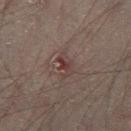Clinical impression: Captured during whole-body skin photography for melanoma surveillance; the lesion was not biopsied. Clinical summary: About 3.5 mm across. A male subject, aged around 50. On the right thigh. A 15 mm close-up extracted from a 3D total-body photography capture.